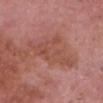Q: Was a biopsy performed?
A: catalogued during a skin exam; not biopsied
Q: Who is the patient?
A: male, roughly 65 years of age
Q: Where on the body is the lesion?
A: the chest
Q: What is the imaging modality?
A: ~15 mm tile from a whole-body skin photo